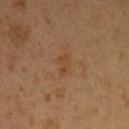biopsy_status: not biopsied; imaged during a skin examination
patient:
  sex: female
  age_approx: 20
image:
  source: total-body photography crop
  field_of_view_mm: 15
site: left upper arm